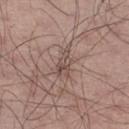biopsy status: catalogued during a skin exam; not biopsied
image: ~15 mm crop, total-body skin-cancer survey
patient: male, approximately 55 years of age
anatomic site: the left thigh